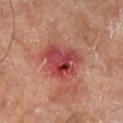No biopsy was performed on this lesion — it was imaged during a full skin examination and was not determined to be concerning. The lesion's longest dimension is about 6 mm. An algorithmic analysis of the crop reported a lesion area of about 19 mm², an eccentricity of roughly 0.65, and a shape-asymmetry score of about 0.25 (0 = symmetric). It also reported an average lesion color of about L≈48 a*≈34 b*≈27 (CIELAB) and a normalized border contrast of about 9. And it measured a color-variation rating of about 8.5/10. A close-up tile cropped from a whole-body skin photograph, about 15 mm across. On the right lower leg. This is a cross-polarized tile.